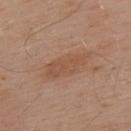Q: Was a biopsy performed?
A: no biopsy performed (imaged during a skin exam)
Q: How was this image acquired?
A: total-body-photography crop, ~15 mm field of view
Q: Where on the body is the lesion?
A: the back
Q: Who is the patient?
A: male, roughly 55 years of age
Q: Automated lesion metrics?
A: a lesion color around L≈52 a*≈21 b*≈31 in CIELAB and a normalized lesion–skin contrast near 5.5; border irregularity of about 3 on a 0–10 scale, a within-lesion color-variation index near 2/10, and peripheral color asymmetry of about 1